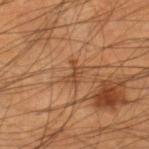{
  "biopsy_status": "not biopsied; imaged during a skin examination",
  "lighting": "cross-polarized",
  "site": "leg",
  "image": {
    "source": "total-body photography crop",
    "field_of_view_mm": 15
  },
  "patient": {
    "sex": "male",
    "age_approx": 45
  },
  "lesion_size": {
    "long_diameter_mm_approx": 2.5
  }
}